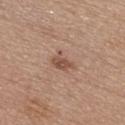This is a white-light tile. Approximately 3 mm at its widest. On the chest. A female subject aged 63–67. A 15 mm close-up extracted from a 3D total-body photography capture.